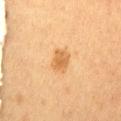Assessment: Part of a total-body skin-imaging series; this lesion was reviewed on a skin check and was not flagged for biopsy. Clinical summary: The subject is a female aged 48 to 52. Imaged with cross-polarized lighting. A close-up tile cropped from a whole-body skin photograph, about 15 mm across. An algorithmic analysis of the crop reported a border-irregularity index near 1.5/10, a color-variation rating of about 2/10, and a peripheral color-asymmetry measure near 1. The lesion is on the lower back.